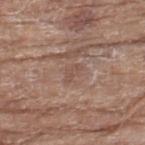{"biopsy_status": "not biopsied; imaged during a skin examination", "lesion_size": {"long_diameter_mm_approx": 2.5}, "site": "left thigh", "automated_metrics": {"cielab_L": 50, "cielab_a": 18, "cielab_b": 25, "vs_skin_darker_L": 6.0}, "image": {"source": "total-body photography crop", "field_of_view_mm": 15}, "patient": {"sex": "female", "age_approx": 75}}